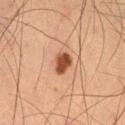biopsy_status: not biopsied; imaged during a skin examination
lesion_size:
  long_diameter_mm_approx: 2.5
patient:
  sex: male
  age_approx: 50
lighting: cross-polarized
image:
  source: total-body photography crop
  field_of_view_mm: 15
site: right thigh